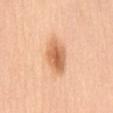Impression:
Captured during whole-body skin photography for melanoma surveillance; the lesion was not biopsied.
Background:
On the chest. Approximately 5.5 mm at its widest. A 15 mm close-up extracted from a 3D total-body photography capture. The total-body-photography lesion software estimated a lesion area of about 11 mm², an outline eccentricity of about 0.85 (0 = round, 1 = elongated), and a shape-asymmetry score of about 0.2 (0 = symmetric). A female subject, aged approximately 40.